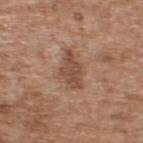Impression:
Imaged during a routine full-body skin examination; the lesion was not biopsied and no histopathology is available.
Acquisition and patient details:
A region of skin cropped from a whole-body photographic capture, roughly 15 mm wide. A male patient, approximately 65 years of age. The lesion's longest dimension is about 5 mm. Located on the upper back.